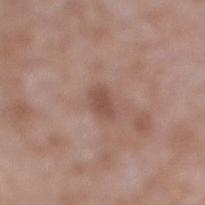biopsy status: no biopsy performed (imaged during a skin exam) | image source: ~15 mm tile from a whole-body skin photo | image-analysis metrics: a footprint of about 4.5 mm², an outline eccentricity of about 0.7 (0 = round, 1 = elongated), and two-axis asymmetry of about 0.2; a lesion color around L≈50 a*≈19 b*≈24 in CIELAB, about 9 CIELAB-L* units darker than the surrounding skin, and a normalized border contrast of about 6.5; border irregularity of about 1.5 on a 0–10 scale, a within-lesion color-variation index near 2/10, and radial color variation of about 1 | patient: male, in their 60s | site: the right lower leg.